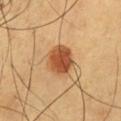No biopsy was performed on this lesion — it was imaged during a full skin examination and was not determined to be concerning. About 4 mm across. A male subject, aged 58 to 62. Imaged with cross-polarized lighting. The lesion is on the chest. This image is a 15 mm lesion crop taken from a total-body photograph.